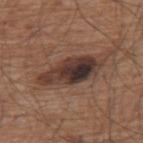Clinical impression: The lesion was photographed on a routine skin check and not biopsied; there is no pathology result. Clinical summary: A region of skin cropped from a whole-body photographic capture, roughly 15 mm wide. The subject is a male approximately 75 years of age. From the upper back.